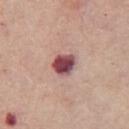{
  "biopsy_status": "not biopsied; imaged during a skin examination",
  "site": "chest",
  "lighting": "white-light",
  "image": {
    "source": "total-body photography crop",
    "field_of_view_mm": 15
  },
  "patient": {
    "sex": "female",
    "age_approx": 70
  },
  "automated_metrics": {
    "area_mm2_approx": 6.5,
    "color_variation_0_10": 5.0,
    "peripheral_color_asymmetry": 1.5,
    "lesion_detection_confidence_0_100": 100
  }
}